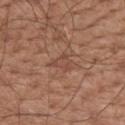Case summary:
– workup — total-body-photography surveillance lesion; no biopsy
– image source — ~15 mm tile from a whole-body skin photo
– illumination — white-light illumination
– anatomic site — the mid back
– patient — male, aged approximately 75